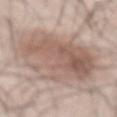<lesion>
<biopsy_status>not biopsied; imaged during a skin examination</biopsy_status>
<patient>
  <sex>male</sex>
  <age_approx>55</age_approx>
</patient>
<lighting>white-light</lighting>
<image>
  <source>total-body photography crop</source>
  <field_of_view_mm>15</field_of_view_mm>
</image>
<site>front of the torso</site>
</lesion>